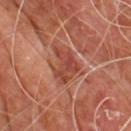No biopsy was performed on this lesion — it was imaged during a full skin examination and was not determined to be concerning. A 15 mm crop from a total-body photograph taken for skin-cancer surveillance. A male subject aged 58 to 62. The lesion is on the upper back.